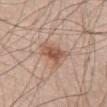- follow-up — catalogued during a skin exam; not biopsied
- location — the back
- subject — male, roughly 35 years of age
- image — total-body-photography crop, ~15 mm field of view
- automated lesion analysis — a lesion area of about 8 mm² and an eccentricity of roughly 0.9; an average lesion color of about L≈55 a*≈21 b*≈29 (CIELAB), about 11 CIELAB-L* units darker than the surrounding skin, and a lesion-to-skin contrast of about 8 (normalized; higher = more distinct); a border-irregularity rating of about 4.5/10 and a within-lesion color-variation index near 4/10
- tile lighting — white-light illumination